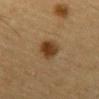workup: imaged on a skin check; not biopsied
tile lighting: cross-polarized
location: the abdomen
size: ≈3.5 mm
TBP lesion metrics: an area of roughly 7.5 mm², an outline eccentricity of about 0.7 (0 = round, 1 = elongated), and a shape-asymmetry score of about 0.2 (0 = symmetric); a nevus-likeness score of about 100/100 and a detector confidence of about 100 out of 100 that the crop contains a lesion
image: ~15 mm tile from a whole-body skin photo
subject: male, approximately 85 years of age or older